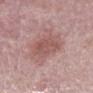* image-analysis metrics — a footprint of about 12 mm², a shape eccentricity near 0.65, and a shape-asymmetry score of about 0.15 (0 = symmetric); a mean CIELAB color near L≈54 a*≈22 b*≈22, a lesion–skin lightness drop of about 9, and a normalized border contrast of about 6.5; a border-irregularity rating of about 1.5/10, a color-variation rating of about 3/10, and peripheral color asymmetry of about 1; a classifier nevus-likeness of about 20/100 and a lesion-detection confidence of about 100/100
* image source — ~15 mm tile from a whole-body skin photo
* illumination — white-light illumination
* anatomic site — the mid back
* diameter — ≈4.5 mm
* subject — male, aged 73–77